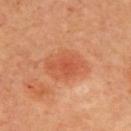Q: Automated lesion metrics?
A: a shape-asymmetry score of about 0.2 (0 = symmetric)
Q: Lesion location?
A: the back
Q: How was this image acquired?
A: ~15 mm crop, total-body skin-cancer survey
Q: How large is the lesion?
A: ≈5 mm
Q: What are the patient's age and sex?
A: male, approximately 65 years of age